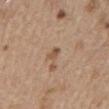No biopsy was performed on this lesion — it was imaged during a full skin examination and was not determined to be concerning. Cropped from a total-body skin-imaging series; the visible field is about 15 mm. On the mid back. About 2.5 mm across. The subject is a male aged 68–72. The tile uses white-light illumination. An algorithmic analysis of the crop reported a mean CIELAB color near L≈53 a*≈17 b*≈31, a lesion–skin lightness drop of about 9, and a lesion-to-skin contrast of about 7 (normalized; higher = more distinct). The software also gave a lesion-detection confidence of about 100/100.